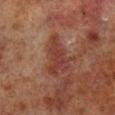Q: Was a biopsy performed?
A: no biopsy performed (imaged during a skin exam)
Q: Automated lesion metrics?
A: an area of roughly 9.5 mm², a shape eccentricity near 0.9, and two-axis asymmetry of about 0.45; a border-irregularity rating of about 6/10, a within-lesion color-variation index near 3/10, and peripheral color asymmetry of about 1.5; a nevus-likeness score of about 0/100
Q: What is the imaging modality?
A: ~15 mm tile from a whole-body skin photo
Q: What are the patient's age and sex?
A: male, aged around 70
Q: What is the anatomic site?
A: the right lower leg
Q: How was the tile lit?
A: cross-polarized illumination